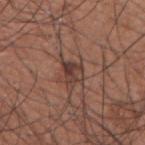Impression:
Recorded during total-body skin imaging; not selected for excision or biopsy.
Acquisition and patient details:
The lesion is located on the upper back. A male patient in their mid- to late 30s. Imaged with white-light lighting. A region of skin cropped from a whole-body photographic capture, roughly 15 mm wide. The recorded lesion diameter is about 3 mm.